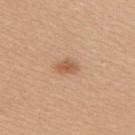Impression:
Recorded during total-body skin imaging; not selected for excision or biopsy.
Image and clinical context:
The total-body-photography lesion software estimated a mean CIELAB color near L≈57 a*≈21 b*≈35 and a lesion–skin lightness drop of about 10. A roughly 15 mm field-of-view crop from a total-body skin photograph. Located on the right upper arm. The tile uses white-light illumination. A female patient in their 40s.